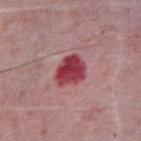| feature | finding |
|---|---|
| workup | imaged on a skin check; not biopsied |
| lesion diameter | ≈3.5 mm |
| image source | 15 mm crop, total-body photography |
| lighting | white-light |
| patient | male, in their mid- to late 70s |
| body site | the abdomen |